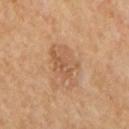Findings:
- workup: no biopsy performed (imaged during a skin exam)
- body site: the mid back
- lesion diameter: about 5.5 mm
- lighting: cross-polarized illumination
- subject: male, about 65 years old
- image source: ~15 mm crop, total-body skin-cancer survey